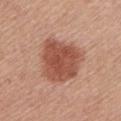Q: Was a biopsy performed?
A: imaged on a skin check; not biopsied
Q: What lighting was used for the tile?
A: white-light
Q: Lesion size?
A: ~5.5 mm (longest diameter)
Q: Lesion location?
A: the front of the torso
Q: Patient demographics?
A: female, in their mid- to late 50s
Q: How was this image acquired?
A: ~15 mm crop, total-body skin-cancer survey